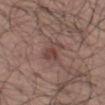Captured during whole-body skin photography for melanoma surveillance; the lesion was not biopsied. Located on the right leg. A region of skin cropped from a whole-body photographic capture, roughly 15 mm wide. The subject is a male in their 70s.